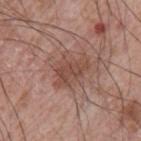Recorded during total-body skin imaging; not selected for excision or biopsy. The tile uses white-light illumination. A close-up tile cropped from a whole-body skin photograph, about 15 mm across. Approximately 5 mm at its widest. The lesion is on the arm. Automated tile analysis of the lesion measured a lesion area of about 10 mm², an outline eccentricity of about 0.75 (0 = round, 1 = elongated), and two-axis asymmetry of about 0.4. And it measured a lesion color around L≈48 a*≈21 b*≈26 in CIELAB, about 8 CIELAB-L* units darker than the surrounding skin, and a normalized border contrast of about 6.5. And it measured a border-irregularity index near 4.5/10 and a peripheral color-asymmetry measure near 1. A male patient approximately 65 years of age.